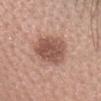Impression: Part of a total-body skin-imaging series; this lesion was reviewed on a skin check and was not flagged for biopsy. Background: A 15 mm close-up extracted from a 3D total-body photography capture. A female subject aged 53–57. From the head or neck. Captured under white-light illumination. Automated image analysis of the tile measured an area of roughly 16 mm² and a shape eccentricity near 0.65. The analysis additionally found a nevus-likeness score of about 55/100.